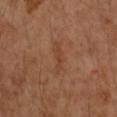Recorded during total-body skin imaging; not selected for excision or biopsy.
On the right forearm.
The subject is a female aged approximately 60.
A lesion tile, about 15 mm wide, cut from a 3D total-body photograph.